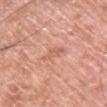follow-up: no biopsy performed (imaged during a skin exam) | lesion size: ≈3 mm | imaging modality: total-body-photography crop, ~15 mm field of view | subject: male, aged approximately 60 | site: the front of the torso | image-analysis metrics: an area of roughly 3.5 mm², a shape eccentricity near 0.85, and a symmetry-axis asymmetry near 0.4; internal color variation of about 1.5 on a 0–10 scale.